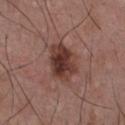{
  "biopsy_status": "not biopsied; imaged during a skin examination",
  "lesion_size": {
    "long_diameter_mm_approx": 5.0
  },
  "site": "chest",
  "image": {
    "source": "total-body photography crop",
    "field_of_view_mm": 15
  },
  "lighting": "white-light",
  "automated_metrics": {
    "area_mm2_approx": 13.0,
    "eccentricity": 0.7,
    "shape_asymmetry": 0.2,
    "cielab_L": 37,
    "cielab_a": 21,
    "cielab_b": 23,
    "vs_skin_darker_L": 12.0,
    "vs_skin_contrast_norm": 10.0,
    "border_irregularity_0_10": 2.0,
    "color_variation_0_10": 6.5,
    "peripheral_color_asymmetry": 2.0
  },
  "patient": {
    "sex": "male",
    "age_approx": 40
  }
}